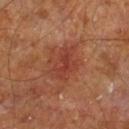biopsy_status: not biopsied; imaged during a skin examination